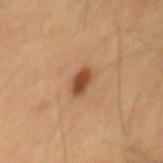Impression: The lesion was tiled from a total-body skin photograph and was not biopsied. Clinical summary: Automated image analysis of the tile measured an outline eccentricity of about 0.85 (0 = round, 1 = elongated). The software also gave a lesion color around L≈41 a*≈20 b*≈31 in CIELAB, a lesion–skin lightness drop of about 12, and a normalized border contrast of about 10. And it measured a border-irregularity index near 2/10, internal color variation of about 3 on a 0–10 scale, and radial color variation of about 1. And it measured a detector confidence of about 100 out of 100 that the crop contains a lesion. The lesion's longest dimension is about 3 mm. The tile uses cross-polarized illumination. The patient is a male aged approximately 60. A 15 mm close-up tile from a total-body photography series done for melanoma screening.